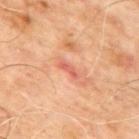This lesion was catalogued during total-body skin photography and was not selected for biopsy.
A male patient, aged 63 to 67.
Cropped from a total-body skin-imaging series; the visible field is about 15 mm.
The lesion is on the upper back.
Imaged with cross-polarized lighting.